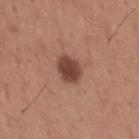biopsy_status: not biopsied; imaged during a skin examination
patient:
  sex: male
  age_approx: 65
image:
  source: total-body photography crop
  field_of_view_mm: 15
lighting: white-light
site: mid back
automated_metrics:
  area_mm2_approx: 7.0
  eccentricity: 0.7
  shape_asymmetry: 0.15
  cielab_L: 44
  cielab_a: 22
  cielab_b: 25
  vs_skin_darker_L: 14.0
lesion_size:
  long_diameter_mm_approx: 3.5